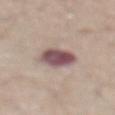Case summary:
* workup · no biopsy performed (imaged during a skin exam)
* lesion diameter · about 4 mm
* anatomic site · the abdomen
* patient · male, aged around 65
* imaging modality · ~15 mm crop, total-body skin-cancer survey